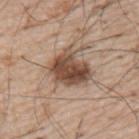Imaged during a routine full-body skin examination; the lesion was not biopsied and no histopathology is available. A male subject in their mid-50s. A roughly 15 mm field-of-view crop from a total-body skin photograph. About 4.5 mm across.